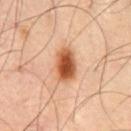Imaged during a routine full-body skin examination; the lesion was not biopsied and no histopathology is available. Measured at roughly 4 mm in maximum diameter. Automated image analysis of the tile measured roughly 18 lightness units darker than nearby skin and a lesion-to-skin contrast of about 12 (normalized; higher = more distinct). And it measured border irregularity of about 1 on a 0–10 scale and radial color variation of about 2. On the chest. A male subject about 55 years old. The tile uses cross-polarized illumination. A 15 mm close-up tile from a total-body photography series done for melanoma screening.